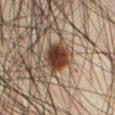biopsy status — imaged on a skin check; not biopsied
illumination — cross-polarized illumination
image — total-body-photography crop, ~15 mm field of view
image-analysis metrics — a footprint of about 9.5 mm² and two-axis asymmetry of about 0.15; border irregularity of about 2 on a 0–10 scale, internal color variation of about 4.5 on a 0–10 scale, and a peripheral color-asymmetry measure near 1.5; a detector confidence of about 100 out of 100 that the crop contains a lesion
site — the abdomen
lesion size — ≈4 mm
subject — male, aged 43 to 47